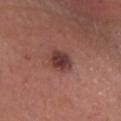Case summary:
- workup: total-body-photography surveillance lesion; no biopsy
- TBP lesion metrics: a mean CIELAB color near L≈38 a*≈23 b*≈22, about 12 CIELAB-L* units darker than the surrounding skin, and a normalized lesion–skin contrast near 10; a border-irregularity index near 1.5/10, a color-variation rating of about 4/10, and peripheral color asymmetry of about 1.5
- illumination: white-light illumination
- lesion size: about 3 mm
- image source: ~15 mm crop, total-body skin-cancer survey
- anatomic site: the head or neck
- patient: male, in their mid- to late 50s